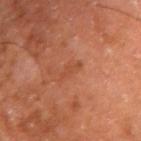Q: Was this lesion biopsied?
A: imaged on a skin check; not biopsied
Q: How was the tile lit?
A: cross-polarized illumination
Q: What did automated image analysis measure?
A: a border-irregularity index near 4.5/10, a color-variation rating of about 0/10, and a peripheral color-asymmetry measure near 0; a classifier nevus-likeness of about 0/100
Q: What is the lesion's diameter?
A: ~2.5 mm (longest diameter)
Q: Patient demographics?
A: in their mid- to late 60s
Q: What is the anatomic site?
A: the right upper arm
Q: What is the imaging modality?
A: ~15 mm crop, total-body skin-cancer survey